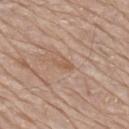{"biopsy_status": "not biopsied; imaged during a skin examination"}